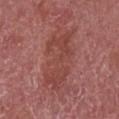notes=no biopsy performed (imaged during a skin exam); subject=female, about 60 years old; lesion diameter=≈7 mm; anatomic site=the right forearm; image source=15 mm crop, total-body photography.